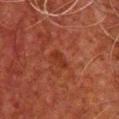Part of a total-body skin-imaging series; this lesion was reviewed on a skin check and was not flagged for biopsy. A male patient approximately 60 years of age. On the chest. Cropped from a total-body skin-imaging series; the visible field is about 15 mm.